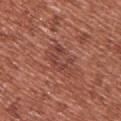{"biopsy_status": "not biopsied; imaged during a skin examination", "lighting": "white-light", "site": "back", "lesion_size": {"long_diameter_mm_approx": 4.5}, "patient": {"sex": "female", "age_approx": 40}, "automated_metrics": {"cielab_L": 43, "cielab_a": 26, "cielab_b": 27, "vs_skin_contrast_norm": 5.5}, "image": {"source": "total-body photography crop", "field_of_view_mm": 15}}